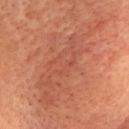{"biopsy_status": "not biopsied; imaged during a skin examination", "image": {"source": "total-body photography crop", "field_of_view_mm": 15}, "lighting": "cross-polarized", "lesion_size": {"long_diameter_mm_approx": 12.5}, "site": "head or neck", "automated_metrics": {"area_mm2_approx": 43.0, "eccentricity": 0.9, "shape_asymmetry": 0.4, "cielab_L": 47, "cielab_a": 25, "cielab_b": 29, "vs_skin_darker_L": 6.0, "vs_skin_contrast_norm": 5.0, "border_irregularity_0_10": 7.5, "color_variation_0_10": 3.5}, "patient": {"sex": "female", "age_approx": 60}}